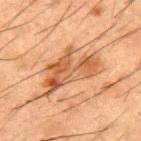Impression:
This lesion was catalogued during total-body skin photography and was not selected for biopsy.
Image and clinical context:
This is a cross-polarized tile. A lesion tile, about 15 mm wide, cut from a 3D total-body photograph. A male subject, aged 48 to 52. Automated tile analysis of the lesion measured a shape eccentricity near 0.85 and a symmetry-axis asymmetry near 0.45. And it measured a border-irregularity rating of about 6/10, internal color variation of about 6.5 on a 0–10 scale, and a peripheral color-asymmetry measure near 2. It also reported a nevus-likeness score of about 10/100 and lesion-presence confidence of about 100/100. The lesion is located on the upper back.